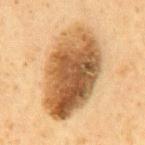biopsy status: total-body-photography surveillance lesion; no biopsy | location: the back | image: 15 mm crop, total-body photography | patient: male, approximately 60 years of age.